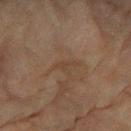biopsy_status: not biopsied; imaged during a skin examination
image:
  source: total-body photography crop
  field_of_view_mm: 15
patient:
  sex: female
  age_approx: 80
site: right forearm